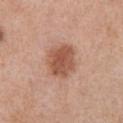The lesion was tiled from a total-body skin photograph and was not biopsied. The total-body-photography lesion software estimated border irregularity of about 2 on a 0–10 scale, a color-variation rating of about 3.5/10, and radial color variation of about 1. The lesion is located on the abdomen. A male subject roughly 70 years of age. The tile uses white-light illumination. This image is a 15 mm lesion crop taken from a total-body photograph. The lesion's longest dimension is about 4.5 mm.